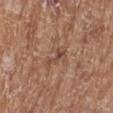Clinical impression:
No biopsy was performed on this lesion — it was imaged during a full skin examination and was not determined to be concerning.
Image and clinical context:
Automated image analysis of the tile measured an area of roughly 4 mm². The analysis additionally found an average lesion color of about L≈47 a*≈20 b*≈29 (CIELAB) and about 8 CIELAB-L* units darker than the surrounding skin. It also reported a detector confidence of about 95 out of 100 that the crop contains a lesion. A 15 mm crop from a total-body photograph taken for skin-cancer surveillance. Located on the left lower leg. The tile uses white-light illumination. The recorded lesion diameter is about 3.5 mm. A female subject, roughly 75 years of age.